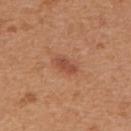Q: Was this lesion biopsied?
A: total-body-photography surveillance lesion; no biopsy
Q: What kind of image is this?
A: total-body-photography crop, ~15 mm field of view
Q: Automated lesion metrics?
A: an area of roughly 4 mm² and an eccentricity of roughly 0.9; radial color variation of about 0.5; lesion-presence confidence of about 100/100
Q: What is the lesion's diameter?
A: about 3 mm
Q: What is the anatomic site?
A: the upper back
Q: Illumination type?
A: white-light illumination
Q: Who is the patient?
A: female, approximately 40 years of age